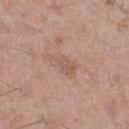Assessment:
Imaged during a routine full-body skin examination; the lesion was not biopsied and no histopathology is available.
Context:
The lesion is located on the left thigh. The tile uses white-light illumination. Approximately 4 mm at its widest. A 15 mm close-up extracted from a 3D total-body photography capture. A male patient, aged around 60.